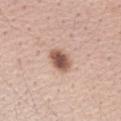No biopsy was performed on this lesion — it was imaged during a full skin examination and was not determined to be concerning. Approximately 3.5 mm at its widest. A female patient in their 40s. Located on the right forearm. Imaged with white-light lighting. This image is a 15 mm lesion crop taken from a total-body photograph.